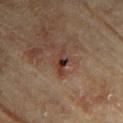Captured during whole-body skin photography for melanoma surveillance; the lesion was not biopsied.
About 3.5 mm across.
Automated image analysis of the tile measured a lesion area of about 4 mm², an outline eccentricity of about 0.9 (0 = round, 1 = elongated), and two-axis asymmetry of about 0.3. And it measured an automated nevus-likeness rating near 0 out of 100 and a lesion-detection confidence of about 100/100.
The lesion is located on the upper back.
This is a cross-polarized tile.
A female subject, approximately 80 years of age.
Cropped from a total-body skin-imaging series; the visible field is about 15 mm.